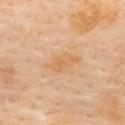The lesion was photographed on a routine skin check and not biopsied; there is no pathology result.
A region of skin cropped from a whole-body photographic capture, roughly 15 mm wide.
On the back.
Longest diameter approximately 4.5 mm.
The lesion-visualizer software estimated an area of roughly 7 mm², an outline eccentricity of about 0.85 (0 = round, 1 = elongated), and a shape-asymmetry score of about 0.25 (0 = symmetric). The analysis additionally found a nevus-likeness score of about 0/100 and a lesion-detection confidence of about 100/100.
Captured under cross-polarized illumination.
The subject is a female approximately 60 years of age.